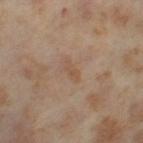This lesion was catalogued during total-body skin photography and was not selected for biopsy. Automated tile analysis of the lesion measured an area of roughly 2.5 mm². The software also gave a border-irregularity rating of about 3.5/10, a color-variation rating of about 0/10, and a peripheral color-asymmetry measure near 0. And it measured a classifier nevus-likeness of about 0/100 and lesion-presence confidence of about 100/100. Captured under cross-polarized illumination. On the left thigh. The subject is a female aged around 55. The lesion's longest dimension is about 2.5 mm. Cropped from a total-body skin-imaging series; the visible field is about 15 mm.